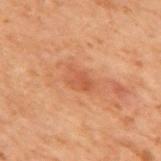biopsy status: catalogued during a skin exam; not biopsied
subject: male, in their 70s
lighting: cross-polarized
location: the mid back
image: ~15 mm crop, total-body skin-cancer survey
lesion size: ~3 mm (longest diameter)